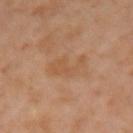Part of a total-body skin-imaging series; this lesion was reviewed on a skin check and was not flagged for biopsy. On the arm. Captured under cross-polarized illumination. A lesion tile, about 15 mm wide, cut from a 3D total-body photograph. The subject is a female aged 38–42.